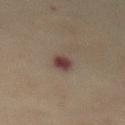Case summary:
* follow-up — no biopsy performed (imaged during a skin exam)
* anatomic site — the front of the torso
* imaging modality — ~15 mm tile from a whole-body skin photo
* illumination — cross-polarized
* subject — female, approximately 70 years of age
* lesion diameter — ≈2.5 mm